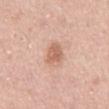| feature | finding |
|---|---|
| follow-up | no biopsy performed (imaged during a skin exam) |
| subject | male, approximately 55 years of age |
| acquisition | 15 mm crop, total-body photography |
| automated metrics | a lesion color around L≈62 a*≈22 b*≈31 in CIELAB, a lesion–skin lightness drop of about 10, and a normalized lesion–skin contrast near 7 |
| illumination | white-light illumination |
| body site | the front of the torso |
| size | ≈2.5 mm |